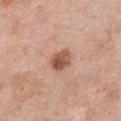A female subject aged around 50. This image is a 15 mm lesion crop taken from a total-body photograph. Captured under white-light illumination. Located on the chest.